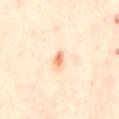This lesion was catalogued during total-body skin photography and was not selected for biopsy. From the mid back. A female patient aged around 40. Cropped from a whole-body photographic skin survey; the tile spans about 15 mm. Measured at roughly 2.5 mm in maximum diameter. Automated tile analysis of the lesion measured a lesion area of about 2.5 mm², an eccentricity of roughly 0.85, and a shape-asymmetry score of about 0.35 (0 = symmetric). The software also gave a border-irregularity index near 3/10, internal color variation of about 1.5 on a 0–10 scale, and a peripheral color-asymmetry measure near 0.5. The software also gave an automated nevus-likeness rating near 85 out of 100 and a lesion-detection confidence of about 100/100.